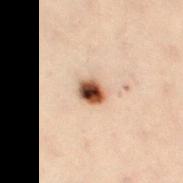Part of a total-body skin-imaging series; this lesion was reviewed on a skin check and was not flagged for biopsy.
This image is a 15 mm lesion crop taken from a total-body photograph.
The subject is a female aged approximately 30.
Located on the left thigh.
Imaged with cross-polarized lighting.